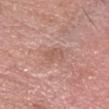Captured during whole-body skin photography for melanoma surveillance; the lesion was not biopsied. Captured under white-light illumination. The patient is a male roughly 70 years of age. The lesion-visualizer software estimated a footprint of about 3.5 mm² and a symmetry-axis asymmetry near 0.2. The analysis additionally found an average lesion color of about L≈56 a*≈22 b*≈27 (CIELAB), a lesion–skin lightness drop of about 7, and a lesion-to-skin contrast of about 5.5 (normalized; higher = more distinct). The lesion is located on the head or neck. The recorded lesion diameter is about 3 mm. A 15 mm crop from a total-body photograph taken for skin-cancer surveillance.